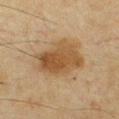biopsy status: no biopsy performed (imaged during a skin exam) | site: the chest | automated lesion analysis: an area of roughly 21 mm², an outline eccentricity of about 0.65 (0 = round, 1 = elongated), and a symmetry-axis asymmetry near 0.2; about 9 CIELAB-L* units darker than the surrounding skin and a normalized border contrast of about 8; a border-irregularity index near 2.5/10 and radial color variation of about 1.5 | patient: male, aged around 65 | lesion diameter: ≈6 mm | illumination: cross-polarized illumination | image: ~15 mm tile from a whole-body skin photo.